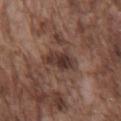follow-up — catalogued during a skin exam; not biopsied
location — the front of the torso
subject — male, aged approximately 75
acquisition — ~15 mm tile from a whole-body skin photo